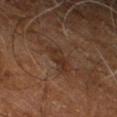Case summary:
* biopsy status · catalogued during a skin exam; not biopsied
* diameter · ~3.5 mm (longest diameter)
* image source · total-body-photography crop, ~15 mm field of view
* anatomic site · the right leg
* subject · male, in their 60s